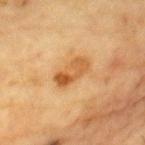follow-up — total-body-photography surveillance lesion; no biopsy | illumination — cross-polarized illumination | subject — male, aged around 85 or older | image source — ~15 mm tile from a whole-body skin photo | site — the chest | image-analysis metrics — a lesion area of about 8 mm², an eccentricity of roughly 0.85, and two-axis asymmetry of about 0.2; about 10 CIELAB-L* units darker than the surrounding skin and a normalized lesion–skin contrast near 7.5; a color-variation rating of about 7/10 and a peripheral color-asymmetry measure near 2.5.